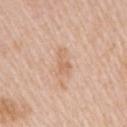workup: total-body-photography surveillance lesion; no biopsy
image source: total-body-photography crop, ~15 mm field of view
patient: female, in their mid- to late 40s
body site: the arm
lesion diameter: ≈3 mm